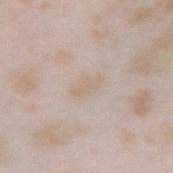Q: Is there a histopathology result?
A: catalogued during a skin exam; not biopsied
Q: How was this image acquired?
A: 15 mm crop, total-body photography
Q: What did automated image analysis measure?
A: lesion-presence confidence of about 80/100
Q: What are the patient's age and sex?
A: female, aged 23–27
Q: Lesion location?
A: the right forearm
Q: What is the lesion's diameter?
A: ~3.5 mm (longest diameter)